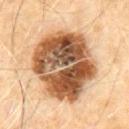Q: Is there a histopathology result?
A: catalogued during a skin exam; not biopsied
Q: Where on the body is the lesion?
A: the front of the torso
Q: How was this image acquired?
A: 15 mm crop, total-body photography
Q: What is the lesion's diameter?
A: about 8.5 mm
Q: What lighting was used for the tile?
A: cross-polarized illumination
Q: Who is the patient?
A: male, approximately 60 years of age